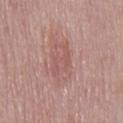Part of a total-body skin-imaging series; this lesion was reviewed on a skin check and was not flagged for biopsy. A male patient aged 73–77. On the mid back. A lesion tile, about 15 mm wide, cut from a 3D total-body photograph.